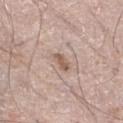Imaged during a routine full-body skin examination; the lesion was not biopsied and no histopathology is available. A male patient approximately 60 years of age. An algorithmic analysis of the crop reported a lesion color around L≈57 a*≈17 b*≈26 in CIELAB and roughly 11 lightness units darker than nearby skin. It also reported a nevus-likeness score of about 10/100 and lesion-presence confidence of about 100/100. A region of skin cropped from a whole-body photographic capture, roughly 15 mm wide. The lesion is on the right lower leg.